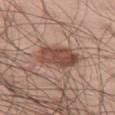{"biopsy_status": "not biopsied; imaged during a skin examination", "site": "left thigh", "patient": {"sex": "male", "age_approx": 55}, "automated_metrics": {"area_mm2_approx": 13.0, "eccentricity": 0.8, "cielab_L": 48, "cielab_a": 21, "cielab_b": 27, "vs_skin_darker_L": 12.0, "vs_skin_contrast_norm": 9.0, "nevus_likeness_0_100": 75}, "lesion_size": {"long_diameter_mm_approx": 5.0}, "image": {"source": "total-body photography crop", "field_of_view_mm": 15}}